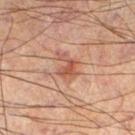patient — male, aged around 60
illumination — cross-polarized illumination
automated metrics — a lesion area of about 4.5 mm² and a shape eccentricity near 0.65; a lesion color around L≈40 a*≈20 b*≈24 in CIELAB, about 8 CIELAB-L* units darker than the surrounding skin, and a normalized lesion–skin contrast near 6.5; border irregularity of about 6.5 on a 0–10 scale and internal color variation of about 1 on a 0–10 scale
diameter — ~2.5 mm (longest diameter)
acquisition — ~15 mm crop, total-body skin-cancer survey
site — the left lower leg